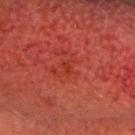{
  "biopsy_status": "not biopsied; imaged during a skin examination",
  "site": "head or neck",
  "image": {
    "source": "total-body photography crop",
    "field_of_view_mm": 15
  },
  "patient": {
    "sex": "male",
    "age_approx": 50
  }
}